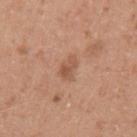| feature | finding |
|---|---|
| follow-up | no biopsy performed (imaged during a skin exam) |
| location | the left upper arm |
| acquisition | total-body-photography crop, ~15 mm field of view |
| patient | female, about 40 years old |